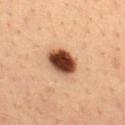follow-up: no biopsy performed (imaged during a skin exam)
body site: the upper back
image source: ~15 mm tile from a whole-body skin photo
patient: male, in their mid- to late 30s